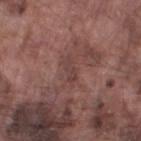Clinical impression:
No biopsy was performed on this lesion — it was imaged during a full skin examination and was not determined to be concerning.
Acquisition and patient details:
Imaged with white-light lighting. The lesion's longest dimension is about 3 mm. A male patient roughly 75 years of age. An algorithmic analysis of the crop reported a shape-asymmetry score of about 0.25 (0 = symmetric). The software also gave an average lesion color of about L≈41 a*≈20 b*≈21 (CIELAB), about 6 CIELAB-L* units darker than the surrounding skin, and a lesion-to-skin contrast of about 5 (normalized; higher = more distinct). The lesion is located on the left thigh. A roughly 15 mm field-of-view crop from a total-body skin photograph.